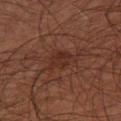The lesion was photographed on a routine skin check and not biopsied; there is no pathology result. This image is a 15 mm lesion crop taken from a total-body photograph. From the left lower leg. The total-body-photography lesion software estimated a lesion area of about 5 mm², an eccentricity of roughly 0.65, and a shape-asymmetry score of about 0.3 (0 = symmetric). And it measured a nevus-likeness score of about 0/100 and a detector confidence of about 100 out of 100 that the crop contains a lesion. A male patient, aged 58 to 62. Approximately 3 mm at its widest.